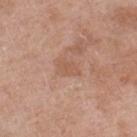<lesion>
  <biopsy_status>not biopsied; imaged during a skin examination</biopsy_status>
  <lighting>white-light</lighting>
  <image>
    <source>total-body photography crop</source>
    <field_of_view_mm>15</field_of_view_mm>
  </image>
  <automated_metrics>
    <nevus_likeness_0_100>0</nevus_likeness_0_100>
    <lesion_detection_confidence_0_100>100</lesion_detection_confidence_0_100>
  </automated_metrics>
  <site>right lower leg</site>
  <lesion_size>
    <long_diameter_mm_approx>3.0</long_diameter_mm_approx>
  </lesion_size>
  <patient>
    <sex>female</sex>
    <age_approx>70</age_approx>
  </patient>
</lesion>